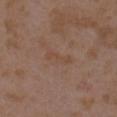Clinical impression: The lesion was tiled from a total-body skin photograph and was not biopsied. Background: Imaged with white-light lighting. The subject is a female approximately 35 years of age. On the left upper arm. This image is a 15 mm lesion crop taken from a total-body photograph.